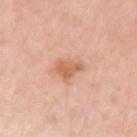biopsy status — imaged on a skin check; not biopsied
body site — the left upper arm
imaging modality — ~15 mm crop, total-body skin-cancer survey
lighting — white-light illumination
automated lesion analysis — an area of roughly 6 mm² and an outline eccentricity of about 0.5 (0 = round, 1 = elongated); a mean CIELAB color near L≈63 a*≈24 b*≈34, roughly 10 lightness units darker than nearby skin, and a normalized border contrast of about 7; an automated nevus-likeness rating near 20 out of 100 and a detector confidence of about 100 out of 100 that the crop contains a lesion
subject — female, aged 38–42
size — about 3 mm